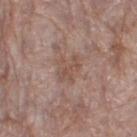Impression:
The lesion was tiled from a total-body skin photograph and was not biopsied.
Background:
A female patient, approximately 85 years of age or older. Located on the leg. This image is a 15 mm lesion crop taken from a total-body photograph.